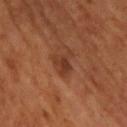notes = imaged on a skin check; not biopsied | site = the upper back | illumination = cross-polarized | size = about 3 mm | patient = male, approximately 65 years of age | image = total-body-photography crop, ~15 mm field of view.